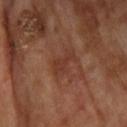Notes:
– subject · male, about 70 years old
– body site · the upper back
– diameter · ~3 mm (longest diameter)
– image-analysis metrics · a lesion area of about 4.5 mm² and a shape eccentricity near 0.85
– image · ~15 mm tile from a whole-body skin photo
– illumination · cross-polarized illumination